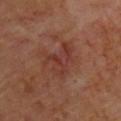Part of a total-body skin-imaging series; this lesion was reviewed on a skin check and was not flagged for biopsy.
Imaged with cross-polarized lighting.
The subject is a male in their 60s.
From the back.
Cropped from a whole-body photographic skin survey; the tile spans about 15 mm.
Approximately 4.5 mm at its widest.